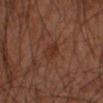Imaged during a routine full-body skin examination; the lesion was not biopsied and no histopathology is available. A male patient, aged 43 to 47. Imaged with cross-polarized lighting. About 3 mm across. This image is a 15 mm lesion crop taken from a total-body photograph. Located on the left forearm.